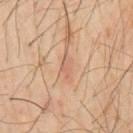notes=imaged on a skin check; not biopsied
body site=the chest
imaging modality=~15 mm tile from a whole-body skin photo
patient=male, approximately 60 years of age